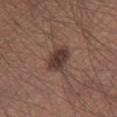- follow-up — catalogued during a skin exam; not biopsied
- patient — male, approximately 45 years of age
- image source — ~15 mm tile from a whole-body skin photo
- site — the leg
- lesion diameter — ≈3.5 mm
- illumination — white-light